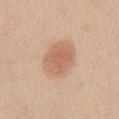Clinical impression: Part of a total-body skin-imaging series; this lesion was reviewed on a skin check and was not flagged for biopsy. Acquisition and patient details: The subject is a female approximately 40 years of age. A 15 mm crop from a total-body photograph taken for skin-cancer surveillance. The tile uses white-light illumination. From the abdomen. Approximately 5 mm at its widest.